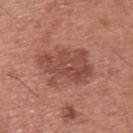<lesion>
<biopsy_status>not biopsied; imaged during a skin examination</biopsy_status>
<lighting>white-light</lighting>
<automated_metrics>
  <area_mm2_approx>23.0</area_mm2_approx>
  <shape_asymmetry>0.25</shape_asymmetry>
  <cielab_L>48</cielab_L>
  <cielab_a>25</cielab_a>
  <cielab_b>27</cielab_b>
  <vs_skin_contrast_norm>7.0</vs_skin_contrast_norm>
</automated_metrics>
<site>upper back</site>
<image>
  <source>total-body photography crop</source>
  <field_of_view_mm>15</field_of_view_mm>
</image>
<patient>
  <sex>male</sex>
  <age_approx>30</age_approx>
</patient>
</lesion>